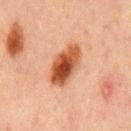Assessment: The lesion was photographed on a routine skin check and not biopsied; there is no pathology result. Context: Approximately 5.5 mm at its widest. A male patient roughly 65 years of age. From the mid back. A close-up tile cropped from a whole-body skin photograph, about 15 mm across. The total-body-photography lesion software estimated an average lesion color of about L≈42 a*≈24 b*≈31 (CIELAB), a lesion–skin lightness drop of about 15, and a lesion-to-skin contrast of about 11.5 (normalized; higher = more distinct). It also reported a border-irregularity rating of about 2/10, a color-variation rating of about 8/10, and radial color variation of about 2.5. The analysis additionally found an automated nevus-likeness rating near 100 out of 100 and a detector confidence of about 100 out of 100 that the crop contains a lesion.